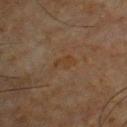workup: no biopsy performed (imaged during a skin exam) | image: total-body-photography crop, ~15 mm field of view | diameter: ≈3.5 mm | body site: the chest | subject: male, in their mid-60s | automated metrics: a shape-asymmetry score of about 0.35 (0 = symmetric); an average lesion color of about L≈32 a*≈15 b*≈27 (CIELAB), roughly 4 lightness units darker than nearby skin, and a lesion-to-skin contrast of about 5.5 (normalized; higher = more distinct).